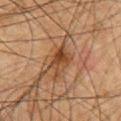Clinical impression: This lesion was catalogued during total-body skin photography and was not selected for biopsy. Acquisition and patient details: Automated tile analysis of the lesion measured internal color variation of about 5.5 on a 0–10 scale and peripheral color asymmetry of about 2. The recorded lesion diameter is about 4 mm. Cropped from a whole-body photographic skin survey; the tile spans about 15 mm. A male subject approximately 50 years of age. On the front of the torso.